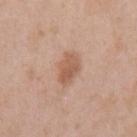Impression:
Imaged during a routine full-body skin examination; the lesion was not biopsied and no histopathology is available.
Context:
The lesion's longest dimension is about 3.5 mm. A 15 mm crop from a total-body photograph taken for skin-cancer surveillance. An algorithmic analysis of the crop reported a lesion–skin lightness drop of about 10 and a normalized border contrast of about 7. It also reported a border-irregularity index near 2/10 and a within-lesion color-variation index near 3/10. The software also gave a nevus-likeness score of about 55/100. From the left upper arm. A female subject approximately 40 years of age.